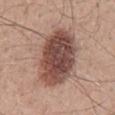Q: Was a biopsy performed?
A: no biopsy performed (imaged during a skin exam)
Q: How was this image acquired?
A: 15 mm crop, total-body photography
Q: Lesion size?
A: ~8.5 mm (longest diameter)
Q: Who is the patient?
A: male, aged 48 to 52
Q: What is the anatomic site?
A: the back
Q: What lighting was used for the tile?
A: white-light illumination